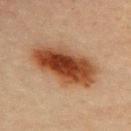Automated image analysis of the tile measured a lesion color around L≈39 a*≈22 b*≈32 in CIELAB, about 16 CIELAB-L* units darker than the surrounding skin, and a normalized border contrast of about 12.5. The software also gave border irregularity of about 2.5 on a 0–10 scale, a color-variation rating of about 8/10, and peripheral color asymmetry of about 2. It also reported a classifier nevus-likeness of about 100/100. This is a cross-polarized tile. The patient is a female roughly 45 years of age. The recorded lesion diameter is about 8.5 mm. A region of skin cropped from a whole-body photographic capture, roughly 15 mm wide. The lesion is located on the upper back.